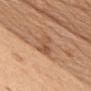Part of a total-body skin-imaging series; this lesion was reviewed on a skin check and was not flagged for biopsy. A female subject, aged approximately 60. A roughly 15 mm field-of-view crop from a total-body skin photograph. Measured at roughly 4 mm in maximum diameter. On the chest. This is a white-light tile.